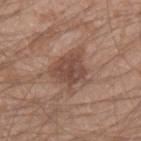Clinical impression:
Part of a total-body skin-imaging series; this lesion was reviewed on a skin check and was not flagged for biopsy.
Clinical summary:
The subject is a male aged 18 to 22. A 15 mm crop from a total-body photograph taken for skin-cancer surveillance. From the left forearm. The lesion's longest dimension is about 5 mm. Imaged with white-light lighting.